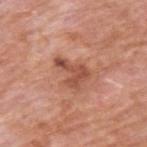Part of a total-body skin-imaging series; this lesion was reviewed on a skin check and was not flagged for biopsy. The subject is a male in their 60s. About 4.5 mm across. Captured under white-light illumination. This image is a 15 mm lesion crop taken from a total-body photograph. From the upper back.